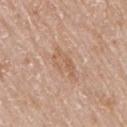Assessment: Imaged during a routine full-body skin examination; the lesion was not biopsied and no histopathology is available. Context: A female subject, aged 83 or older. An algorithmic analysis of the crop reported border irregularity of about 3.5 on a 0–10 scale, a within-lesion color-variation index near 3/10, and a peripheral color-asymmetry measure near 1. It also reported a nevus-likeness score of about 0/100. The lesion's longest dimension is about 4 mm. On the arm. This is a white-light tile. A 15 mm crop from a total-body photograph taken for skin-cancer surveillance.